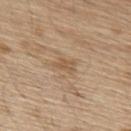Impression: The lesion was photographed on a routine skin check and not biopsied; there is no pathology result. Acquisition and patient details: A male subject aged 68 to 72. Cropped from a total-body skin-imaging series; the visible field is about 15 mm. From the upper back.